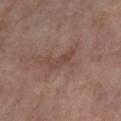notes: total-body-photography surveillance lesion; no biopsy | patient: female, aged around 55 | lighting: cross-polarized | image-analysis metrics: a lesion area of about 4.5 mm² and a shape-asymmetry score of about 0.55 (0 = symmetric); a border-irregularity index near 6/10, a color-variation rating of about 1/10, and radial color variation of about 0 | location: the left lower leg | diameter: ≈3.5 mm | image: ~15 mm tile from a whole-body skin photo.